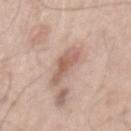<record>
<biopsy_status>not biopsied; imaged during a skin examination</biopsy_status>
<patient>
  <sex>male</sex>
  <age_approx>50</age_approx>
</patient>
<lesion_size>
  <long_diameter_mm_approx>5.0</long_diameter_mm_approx>
</lesion_size>
<image>
  <source>total-body photography crop</source>
  <field_of_view_mm>15</field_of_view_mm>
</image>
<automated_metrics>
  <area_mm2_approx>7.5</area_mm2_approx>
  <eccentricity>0.9</eccentricity>
  <shape_asymmetry>0.35</shape_asymmetry>
  <cielab_L>59</cielab_L>
  <cielab_a>20</cielab_a>
  <cielab_b>26</cielab_b>
  <vs_skin_darker_L>11.0</vs_skin_darker_L>
  <vs_skin_contrast_norm>7.0</vs_skin_contrast_norm>
  <border_irregularity_0_10>4.5</border_irregularity_0_10>
  <color_variation_0_10>3.5</color_variation_0_10>
  <peripheral_color_asymmetry>1.0</peripheral_color_asymmetry>
  <nevus_likeness_0_100>15</nevus_likeness_0_100>
  <lesion_detection_confidence_0_100>100</lesion_detection_confidence_0_100>
</automated_metrics>
<site>mid back</site>
<lighting>white-light</lighting>
</record>